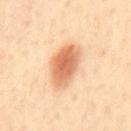This lesion was catalogued during total-body skin photography and was not selected for biopsy. The subject is a male aged 28 to 32. Located on the mid back. A 15 mm crop from a total-body photograph taken for skin-cancer surveillance.